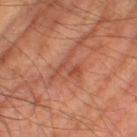Notes:
* notes — total-body-photography surveillance lesion; no biopsy
* illumination — cross-polarized illumination
* image — 15 mm crop, total-body photography
* image-analysis metrics — a lesion area of about 5 mm², a shape eccentricity near 0.55, and two-axis asymmetry of about 0.7; a lesion color around L≈48 a*≈27 b*≈31 in CIELAB, a lesion–skin lightness drop of about 7, and a normalized lesion–skin contrast near 5; a border-irregularity rating of about 10/10, a color-variation rating of about 2/10, and radial color variation of about 0.5
* subject — male, aged approximately 65
* site — the leg